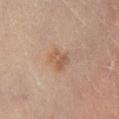image: ~15 mm crop, total-body skin-cancer survey | patient: female, approximately 60 years of age | automated metrics: an area of roughly 5.5 mm² and a shape-asymmetry score of about 0.3 (0 = symmetric); a color-variation rating of about 3/10 and radial color variation of about 1; a nevus-likeness score of about 5/100 and lesion-presence confidence of about 100/100 | body site: the right lower leg | lesion size: about 3 mm | lighting: cross-polarized.